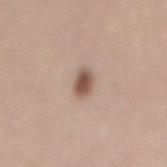Q: Was a biopsy performed?
A: imaged on a skin check; not biopsied
Q: Lesion location?
A: the lower back
Q: How was this image acquired?
A: ~15 mm crop, total-body skin-cancer survey
Q: What are the patient's age and sex?
A: male, approximately 45 years of age
Q: How was the tile lit?
A: white-light illumination